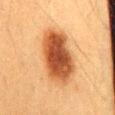The lesion is located on the lower back. A male subject, in their mid- to late 30s. Automated image analysis of the tile measured about 15 CIELAB-L* units darker than the surrounding skin and a normalized border contrast of about 11.5. And it measured border irregularity of about 1.5 on a 0–10 scale, a within-lesion color-variation index near 6.5/10, and radial color variation of about 2. It also reported a nevus-likeness score of about 100/100 and a detector confidence of about 100 out of 100 that the crop contains a lesion. A 15 mm crop from a total-body photograph taken for skin-cancer surveillance. This is a cross-polarized tile. Measured at roughly 7.5 mm in maximum diameter.